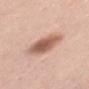acquisition: ~15 mm crop, total-body skin-cancer survey | body site: the back | tile lighting: white-light | subject: male, aged around 55 | lesion size: about 5 mm.